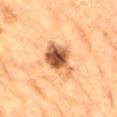Q: Was a biopsy performed?
A: catalogued during a skin exam; not biopsied
Q: How was this image acquired?
A: 15 mm crop, total-body photography
Q: Lesion size?
A: ≈5 mm
Q: How was the tile lit?
A: cross-polarized illumination
Q: Where on the body is the lesion?
A: the lower back
Q: Who is the patient?
A: male, aged 83 or over